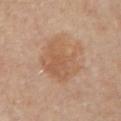biopsy_status: not biopsied; imaged during a skin examination
image:
  source: total-body photography crop
  field_of_view_mm: 15
lighting: cross-polarized
site: left upper arm
lesion_size:
  long_diameter_mm_approx: 6.5
patient:
  sex: female
  age_approx: 50